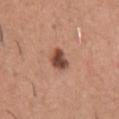About 3 mm across. Cropped from a whole-body photographic skin survey; the tile spans about 15 mm. On the chest. A male patient aged around 45. This is a white-light tile.